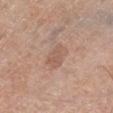This lesion was catalogued during total-body skin photography and was not selected for biopsy.
The recorded lesion diameter is about 3.5 mm.
The lesion is on the leg.
A 15 mm close-up tile from a total-body photography series done for melanoma screening.
A male patient, approximately 70 years of age.